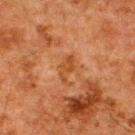Recorded during total-body skin imaging; not selected for excision or biopsy. The lesion is located on the upper back. Cropped from a total-body skin-imaging series; the visible field is about 15 mm. The patient is a male about 60 years old. Imaged with cross-polarized lighting.